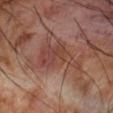| field | value |
|---|---|
| workup | no biopsy performed (imaged during a skin exam) |
| body site | the right forearm |
| image | 15 mm crop, total-body photography |
| TBP lesion metrics | a lesion area of about 12 mm² and a symmetry-axis asymmetry near 0.5; internal color variation of about 5 on a 0–10 scale and a peripheral color-asymmetry measure near 2; an automated nevus-likeness rating near 0 out of 100 and a lesion-detection confidence of about 90/100 |
| patient | male, aged around 70 |
| lesion diameter | ≈6 mm |